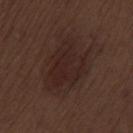Image and clinical context: The total-body-photography lesion software estimated an eccentricity of roughly 0.75. The analysis additionally found a mean CIELAB color near L≈23 a*≈17 b*≈18, a lesion–skin lightness drop of about 5, and a normalized lesion–skin contrast near 7. The software also gave a border-irregularity index near 5/10, internal color variation of about 3.5 on a 0–10 scale, and peripheral color asymmetry of about 1.5. And it measured a classifier nevus-likeness of about 50/100 and a lesion-detection confidence of about 100/100. A male patient, aged 68–72. Imaged with white-light lighting. The lesion is on the left thigh. A lesion tile, about 15 mm wide, cut from a 3D total-body photograph. The recorded lesion diameter is about 6.5 mm.